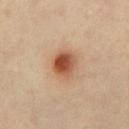Assessment: Recorded during total-body skin imaging; not selected for excision or biopsy. Context: A roughly 15 mm field-of-view crop from a total-body skin photograph. A female subject, approximately 35 years of age. Located on the chest.